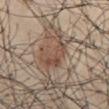<tbp_lesion>
  <biopsy_status>not biopsied; imaged during a skin examination</biopsy_status>
  <patient>
    <sex>male</sex>
    <age_approx>30</age_approx>
  </patient>
  <image>
    <source>total-body photography crop</source>
    <field_of_view_mm>15</field_of_view_mm>
  </image>
  <site>abdomen</site>
  <automated_metrics>
    <area_mm2_approx>9.0</area_mm2_approx>
    <shape_asymmetry>0.5</shape_asymmetry>
    <cielab_L>49</cielab_L>
    <cielab_a>17</cielab_a>
    <cielab_b>27</cielab_b>
    <vs_skin_darker_L>10.0</vs_skin_darker_L>
    <vs_skin_contrast_norm>7.0</vs_skin_contrast_norm>
    <border_irregularity_0_10>6.5</border_irregularity_0_10>
    <color_variation_0_10>4.5</color_variation_0_10>
    <peripheral_color_asymmetry>2.0</peripheral_color_asymmetry>
    <nevus_likeness_0_100>100</nevus_likeness_0_100>
    <lesion_detection_confidence_0_100>65</lesion_detection_confidence_0_100>
  </automated_metrics>
  <lighting>white-light</lighting>
  <lesion_size>
    <long_diameter_mm_approx>5.5</long_diameter_mm_approx>
  </lesion_size>
</tbp_lesion>